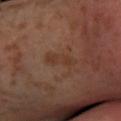biopsy status: total-body-photography surveillance lesion; no biopsy | imaging modality: ~15 mm tile from a whole-body skin photo | image-analysis metrics: a color-variation rating of about 2.5/10 and a peripheral color-asymmetry measure near 1 | lesion diameter: ≈4 mm | patient: male, aged approximately 30 | site: the left leg | tile lighting: cross-polarized illumination.